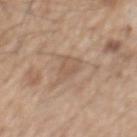Notes:
– follow-up — catalogued during a skin exam; not biopsied
– image-analysis metrics — a footprint of about 6 mm², a shape eccentricity near 0.9, and two-axis asymmetry of about 0.35; a lesion color around L≈56 a*≈16 b*≈29 in CIELAB and a lesion-to-skin contrast of about 5 (normalized; higher = more distinct); a border-irregularity index near 4.5/10, a within-lesion color-variation index near 2/10, and peripheral color asymmetry of about 0.5; a nevus-likeness score of about 0/100
– patient — male, aged 68 to 72
– body site — the chest
– imaging modality — 15 mm crop, total-body photography
– tile lighting — white-light